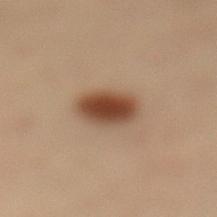This lesion was catalogued during total-body skin photography and was not selected for biopsy.
A male subject, aged 53 to 57.
A roughly 15 mm field-of-view crop from a total-body skin photograph.
The lesion's longest dimension is about 4 mm.
Located on the left lower leg.
This is a cross-polarized tile.
The total-body-photography lesion software estimated a lesion area of about 9.5 mm² and a shape-asymmetry score of about 0.15 (0 = symmetric). It also reported about 13 CIELAB-L* units darker than the surrounding skin and a normalized border contrast of about 11.5. And it measured border irregularity of about 1.5 on a 0–10 scale and internal color variation of about 4 on a 0–10 scale.